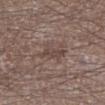Imaged during a routine full-body skin examination; the lesion was not biopsied and no histopathology is available. The total-body-photography lesion software estimated a shape eccentricity near 0.7 and two-axis asymmetry of about 0.3. Measured at roughly 3.5 mm in maximum diameter. From the left lower leg. A male patient in their mid-60s. A 15 mm close-up extracted from a 3D total-body photography capture.